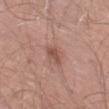Imaged during a routine full-body skin examination; the lesion was not biopsied and no histopathology is available. Cropped from a whole-body photographic skin survey; the tile spans about 15 mm. Imaged with white-light lighting. Located on the left thigh. A male subject, about 70 years old. About 3 mm across.